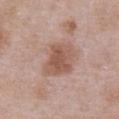This lesion was catalogued during total-body skin photography and was not selected for biopsy. The tile uses white-light illumination. The lesion is on the abdomen. A male patient aged approximately 55. A 15 mm crop from a total-body photograph taken for skin-cancer surveillance.